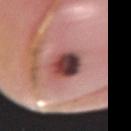workup: no biopsy performed (imaged during a skin exam); anatomic site: the right forearm; patient: male, aged 53–57; lesion diameter: ~4.5 mm (longest diameter); imaging modality: ~15 mm crop, total-body skin-cancer survey.